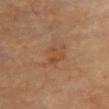The patient is a female in their 70s. This image is a 15 mm lesion crop taken from a total-body photograph. The lesion's longest dimension is about 5 mm. Captured under cross-polarized illumination. On the front of the torso. Automated image analysis of the tile measured about 5 CIELAB-L* units darker than the surrounding skin and a normalized border contrast of about 5. The analysis additionally found a nevus-likeness score of about 0/100 and a detector confidence of about 100 out of 100 that the crop contains a lesion.